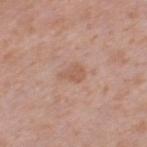Assessment: Imaged during a routine full-body skin examination; the lesion was not biopsied and no histopathology is available. Context: Located on the left thigh. This image is a 15 mm lesion crop taken from a total-body photograph. The subject is a female roughly 40 years of age.